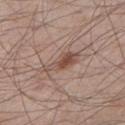Part of a total-body skin-imaging series; this lesion was reviewed on a skin check and was not flagged for biopsy.
A 15 mm close-up tile from a total-body photography series done for melanoma screening.
The total-body-photography lesion software estimated a footprint of about 8 mm², a shape eccentricity near 0.95, and a shape-asymmetry score of about 0.35 (0 = symmetric). And it measured a mean CIELAB color near L≈50 a*≈18 b*≈25 and roughly 10 lightness units darker than nearby skin. It also reported border irregularity of about 4.5 on a 0–10 scale and a peripheral color-asymmetry measure near 1.5.
The tile uses white-light illumination.
The recorded lesion diameter is about 5.5 mm.
The lesion is located on the left lower leg.
A male patient aged approximately 60.